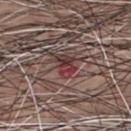Notes:
- biopsy status · no biopsy performed (imaged during a skin exam)
- automated metrics · a lesion area of about 5 mm² and a shape eccentricity near 0.65; a lesion–skin lightness drop of about 10 and a normalized border contrast of about 8.5; a border-irregularity rating of about 4/10 and peripheral color asymmetry of about 5; a nevus-likeness score of about 0/100
- image · ~15 mm tile from a whole-body skin photo
- location · the chest
- tile lighting · white-light
- diameter · ≈3 mm
- patient · male, about 60 years old